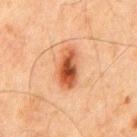The total-body-photography lesion software estimated border irregularity of about 2 on a 0–10 scale, a color-variation rating of about 7.5/10, and radial color variation of about 2. The analysis additionally found lesion-presence confidence of about 100/100.
Imaged with cross-polarized lighting.
A male subject, aged around 65.
The lesion is located on the chest.
A 15 mm crop from a total-body photograph taken for skin-cancer surveillance.
Longest diameter approximately 4.5 mm.